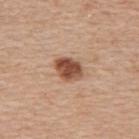subject: female, aged 48–52; size: about 3 mm; image source: 15 mm crop, total-body photography; location: the upper back.